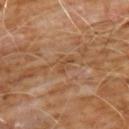imaging modality: 15 mm crop, total-body photography | lesion diameter: ≈2.5 mm | patient: male, aged 58 to 62 | illumination: cross-polarized illumination | location: the chest.